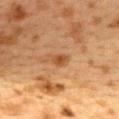notes: imaged on a skin check; not biopsied.